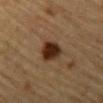• workup — catalogued during a skin exam; not biopsied
• lighting — cross-polarized illumination
• subject — male, about 85 years old or older
• anatomic site — the front of the torso
• image — ~15 mm tile from a whole-body skin photo